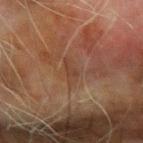Case summary:
- workup — no biopsy performed (imaged during a skin exam)
- illumination — cross-polarized illumination
- acquisition — 15 mm crop, total-body photography
- location — the left forearm
- size — about 3.5 mm
- subject — male, approximately 70 years of age